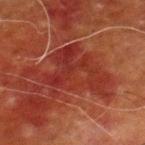Captured during whole-body skin photography for melanoma surveillance; the lesion was not biopsied.